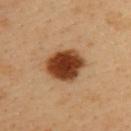Q: Was a biopsy performed?
A: total-body-photography surveillance lesion; no biopsy
Q: Automated lesion metrics?
A: a footprint of about 15 mm² and a symmetry-axis asymmetry near 0.1; border irregularity of about 1 on a 0–10 scale and a color-variation rating of about 5/10
Q: Illumination type?
A: cross-polarized
Q: What are the patient's age and sex?
A: male, roughly 40 years of age
Q: What is the lesion's diameter?
A: ~5 mm (longest diameter)
Q: How was this image acquired?
A: ~15 mm crop, total-body skin-cancer survey
Q: What is the anatomic site?
A: the upper back